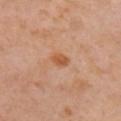Case summary:
- follow-up: total-body-photography surveillance lesion; no biopsy
- image-analysis metrics: an automated nevus-likeness rating near 55 out of 100 and a detector confidence of about 100 out of 100 that the crop contains a lesion
- diameter: about 2.5 mm
- acquisition: total-body-photography crop, ~15 mm field of view
- patient: female, aged 38 to 42
- body site: the right upper arm